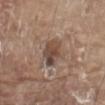Clinical impression:
Captured during whole-body skin photography for melanoma surveillance; the lesion was not biopsied.
Context:
The recorded lesion diameter is about 3.5 mm. A male patient approximately 80 years of age. The lesion is located on the abdomen. Imaged with white-light lighting. Cropped from a total-body skin-imaging series; the visible field is about 15 mm.